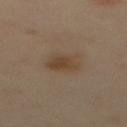biopsy status: catalogued during a skin exam; not biopsied | acquisition: ~15 mm tile from a whole-body skin photo | subject: female, approximately 40 years of age | anatomic site: the upper back.